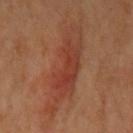This lesion was catalogued during total-body skin photography and was not selected for biopsy. The lesion's longest dimension is about 10.5 mm. Cropped from a whole-body photographic skin survey; the tile spans about 15 mm. The lesion is on the left upper arm. A female patient, aged around 65. Captured under cross-polarized illumination.